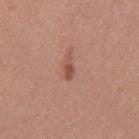Assessment: Part of a total-body skin-imaging series; this lesion was reviewed on a skin check and was not flagged for biopsy. Image and clinical context: Imaged with white-light lighting. The lesion's longest dimension is about 2.5 mm. A lesion tile, about 15 mm wide, cut from a 3D total-body photograph. The subject is a female aged around 50. Located on the mid back.